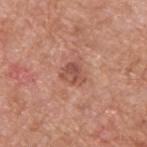This lesion was catalogued during total-body skin photography and was not selected for biopsy.
Approximately 3 mm at its widest.
A male patient, in their 60s.
The tile uses white-light illumination.
Located on the back.
Automated image analysis of the tile measured a lesion color around L≈52 a*≈25 b*≈28 in CIELAB and a normalized border contrast of about 7. The software also gave a detector confidence of about 100 out of 100 that the crop contains a lesion.
A lesion tile, about 15 mm wide, cut from a 3D total-body photograph.